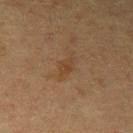Background:
A male patient approximately 60 years of age. This is a cross-polarized tile. An algorithmic analysis of the crop reported a lesion color around L≈36 a*≈15 b*≈29 in CIELAB, a lesion–skin lightness drop of about 5, and a normalized lesion–skin contrast near 5. And it measured a border-irregularity rating of about 2.5/10, internal color variation of about 1 on a 0–10 scale, and peripheral color asymmetry of about 0.5. A roughly 15 mm field-of-view crop from a total-body skin photograph. The lesion's longest dimension is about 2.5 mm.